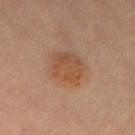Clinical impression: Captured during whole-body skin photography for melanoma surveillance; the lesion was not biopsied. Image and clinical context: Longest diameter approximately 4.5 mm. Captured under cross-polarized illumination. The lesion is located on the left thigh. A roughly 15 mm field-of-view crop from a total-body skin photograph. A female patient aged around 30.